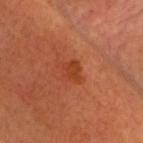Case summary:
- notes: total-body-photography surveillance lesion; no biopsy
- lesion size: ~3 mm (longest diameter)
- tile lighting: cross-polarized illumination
- patient: male, approximately 60 years of age
- body site: the head or neck
- image source: ~15 mm tile from a whole-body skin photo